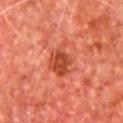{
  "biopsy_status": "not biopsied; imaged during a skin examination",
  "patient": {
    "sex": "male",
    "age_approx": 65
  },
  "site": "chest",
  "image": {
    "source": "total-body photography crop",
    "field_of_view_mm": 15
  },
  "lesion_size": {
    "long_diameter_mm_approx": 4.5
  },
  "lighting": "cross-polarized",
  "automated_metrics": {
    "shape_asymmetry": 0.25,
    "border_irregularity_0_10": 3.0,
    "color_variation_0_10": 3.5,
    "peripheral_color_asymmetry": 1.0
  }
}